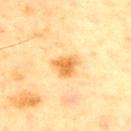<lesion>
<biopsy_status>not biopsied; imaged during a skin examination</biopsy_status>
<image>
  <source>total-body photography crop</source>
  <field_of_view_mm>15</field_of_view_mm>
</image>
<patient>
  <sex>male</sex>
  <age_approx>65</age_approx>
</patient>
<site>front of the torso</site>
</lesion>